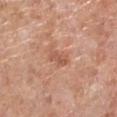Findings:
- notes: catalogued during a skin exam; not biopsied
- size: about 2.5 mm
- tile lighting: white-light illumination
- subject: male, aged approximately 80
- image source: ~15 mm tile from a whole-body skin photo
- body site: the left lower leg